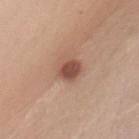Q: Was a biopsy performed?
A: no biopsy performed (imaged during a skin exam)
Q: How large is the lesion?
A: ≈3.5 mm
Q: What kind of image is this?
A: 15 mm crop, total-body photography
Q: What did automated image analysis measure?
A: a lesion area of about 5.5 mm² and an eccentricity of roughly 0.75; a mean CIELAB color near L≈50 a*≈23 b*≈27, about 13 CIELAB-L* units darker than the surrounding skin, and a lesion-to-skin contrast of about 9 (normalized; higher = more distinct); border irregularity of about 2.5 on a 0–10 scale, a within-lesion color-variation index near 3/10, and radial color variation of about 1; an automated nevus-likeness rating near 90 out of 100 and lesion-presence confidence of about 100/100
Q: How was the tile lit?
A: white-light
Q: Patient demographics?
A: female, aged around 40
Q: Lesion location?
A: the chest